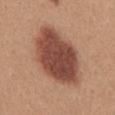{"biopsy_status": "not biopsied; imaged during a skin examination"}